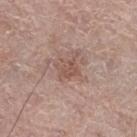Recorded during total-body skin imaging; not selected for excision or biopsy.